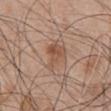Notes:
– notes: imaged on a skin check; not biopsied